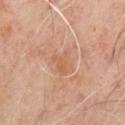biopsy status = catalogued during a skin exam; not biopsied.